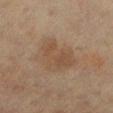Impression: Captured during whole-body skin photography for melanoma surveillance; the lesion was not biopsied.